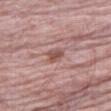Q: Illumination type?
A: white-light
Q: Lesion size?
A: about 2.5 mm
Q: Where on the body is the lesion?
A: the right thigh
Q: What kind of image is this?
A: total-body-photography crop, ~15 mm field of view
Q: Automated lesion metrics?
A: a mean CIELAB color near L≈52 a*≈21 b*≈23, roughly 10 lightness units darker than nearby skin, and a lesion-to-skin contrast of about 7 (normalized; higher = more distinct); a border-irregularity rating of about 2.5/10 and radial color variation of about 1
Q: Patient demographics?
A: male, about 70 years old